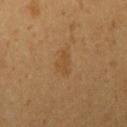Q: Was this lesion biopsied?
A: imaged on a skin check; not biopsied
Q: Automated lesion metrics?
A: border irregularity of about 4.5 on a 0–10 scale and a color-variation rating of about 0.5/10; an automated nevus-likeness rating near 15 out of 100
Q: Lesion size?
A: ~3 mm (longest diameter)
Q: What lighting was used for the tile?
A: cross-polarized
Q: What is the anatomic site?
A: the right upper arm
Q: What are the patient's age and sex?
A: female, approximately 20 years of age
Q: What is the imaging modality?
A: 15 mm crop, total-body photography